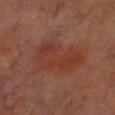notes = catalogued during a skin exam; not biopsied
subject = male, aged 68–72
image = total-body-photography crop, ~15 mm field of view
site = the left lower leg